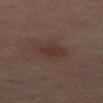follow-up = catalogued during a skin exam; not biopsied
acquisition = 15 mm crop, total-body photography
site = the mid back
patient = male, aged 63 to 67